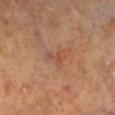The lesion's longest dimension is about 3 mm. The lesion is located on the leg. A 15 mm close-up tile from a total-body photography series done for melanoma screening. The lesion-visualizer software estimated a lesion area of about 3.5 mm², an eccentricity of roughly 0.75, and a symmetry-axis asymmetry near 0.65. The analysis additionally found a border-irregularity rating of about 7/10 and peripheral color asymmetry of about 0. The analysis additionally found a classifier nevus-likeness of about 0/100 and lesion-presence confidence of about 100/100. A female patient roughly 70 years of age.